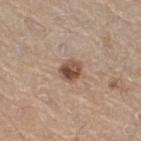<tbp_lesion>
  <biopsy_status>not biopsied; imaged during a skin examination</biopsy_status>
  <image>
    <source>total-body photography crop</source>
    <field_of_view_mm>15</field_of_view_mm>
  </image>
  <lesion_size>
    <long_diameter_mm_approx>2.5</long_diameter_mm_approx>
  </lesion_size>
  <patient>
    <sex>female</sex>
    <age_approx>50</age_approx>
  </patient>
  <site>right thigh</site>
  <lighting>white-light</lighting>
</tbp_lesion>